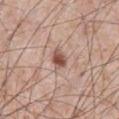biopsy status=no biopsy performed (imaged during a skin exam)
automated lesion analysis=an average lesion color of about L≈52 a*≈22 b*≈26 (CIELAB) and a normalized border contrast of about 9.5
imaging modality=~15 mm crop, total-body skin-cancer survey
anatomic site=the abdomen
patient=male, in their mid-50s
diameter=≈2.5 mm
lighting=white-light